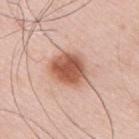biopsy status: catalogued during a skin exam; not biopsied
image source: ~15 mm crop, total-body skin-cancer survey
illumination: white-light illumination
subject: male, roughly 40 years of age
body site: the right upper arm
lesion diameter: about 4.5 mm